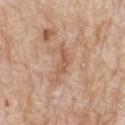Assessment:
No biopsy was performed on this lesion — it was imaged during a full skin examination and was not determined to be concerning.
Acquisition and patient details:
This image is a 15 mm lesion crop taken from a total-body photograph. Approximately 3.5 mm at its widest. The lesion is on the back. The subject is a male aged 83 or older.